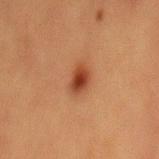The lesion was tiled from a total-body skin photograph and was not biopsied.
A male patient, approximately 40 years of age.
A lesion tile, about 15 mm wide, cut from a 3D total-body photograph.
Approximately 3.5 mm at its widest.
The lesion is on the mid back.
The tile uses cross-polarized illumination.